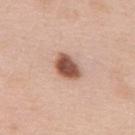Captured during whole-body skin photography for melanoma surveillance; the lesion was not biopsied.
From the upper back.
Cropped from a total-body skin-imaging series; the visible field is about 15 mm.
A male patient approximately 60 years of age.